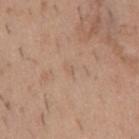Case summary:
- biopsy status — catalogued during a skin exam; not biopsied
- image-analysis metrics — a lesion area of about 1.5 mm², an outline eccentricity of about 0.8 (0 = round, 1 = elongated), and two-axis asymmetry of about 0.25; a lesion color around L≈59 a*≈17 b*≈29 in CIELAB, a lesion–skin lightness drop of about 5, and a normalized lesion–skin contrast near 3.5; a within-lesion color-variation index near 0/10 and a peripheral color-asymmetry measure near 0; an automated nevus-likeness rating near 0 out of 100 and a lesion-detection confidence of about 100/100
- imaging modality — 15 mm crop, total-body photography
- size — about 1.5 mm
- body site — the mid back
- patient — male, aged 38 to 42
- illumination — white-light illumination